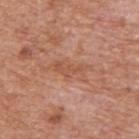Notes:
• biopsy status · total-body-photography surveillance lesion; no biopsy
• lesion diameter · ~5.5 mm (longest diameter)
• acquisition · 15 mm crop, total-body photography
• tile lighting · white-light illumination
• patient · male, roughly 65 years of age
• body site · the upper back
• image-analysis metrics · a border-irregularity index near 8.5/10, internal color variation of about 2 on a 0–10 scale, and radial color variation of about 0.5; an automated nevus-likeness rating near 0 out of 100